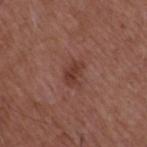Assessment:
The lesion was tiled from a total-body skin photograph and was not biopsied.
Clinical summary:
Captured under white-light illumination. Measured at roughly 2.5 mm in maximum diameter. A male subject, aged 48 to 52. From the upper back. A roughly 15 mm field-of-view crop from a total-body skin photograph.